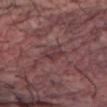Assessment: Captured during whole-body skin photography for melanoma surveillance; the lesion was not biopsied. Acquisition and patient details: The lesion is located on the leg. Cropped from a whole-body photographic skin survey; the tile spans about 15 mm. An algorithmic analysis of the crop reported a lesion area of about 4.5 mm² and two-axis asymmetry of about 0.25. Imaged with white-light lighting. A male subject, roughly 30 years of age.